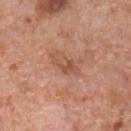Recorded during total-body skin imaging; not selected for excision or biopsy.
The total-body-photography lesion software estimated a border-irregularity index near 6.5/10 and peripheral color asymmetry of about 0. And it measured an automated nevus-likeness rating near 0 out of 100 and lesion-presence confidence of about 100/100.
About 3.5 mm across.
Located on the chest.
A male subject in their 60s.
This is a white-light tile.
A roughly 15 mm field-of-view crop from a total-body skin photograph.